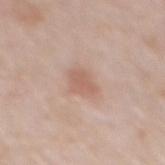| feature | finding |
|---|---|
| notes | total-body-photography surveillance lesion; no biopsy |
| image | 15 mm crop, total-body photography |
| illumination | white-light |
| site | the mid back |
| size | about 2.5 mm |
| patient | male, about 65 years old |